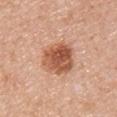– workup: no biopsy performed (imaged during a skin exam)
– illumination: white-light illumination
– lesion diameter: ~5 mm (longest diameter)
– body site: the right upper arm
– image-analysis metrics: an outline eccentricity of about 0.55 (0 = round, 1 = elongated) and a symmetry-axis asymmetry near 0.2; a lesion–skin lightness drop of about 14; a within-lesion color-variation index near 6.5/10; a nevus-likeness score of about 95/100 and a lesion-detection confidence of about 100/100
– patient: female, aged around 50
– image: ~15 mm tile from a whole-body skin photo